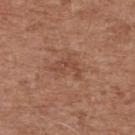Case summary:
* notes · imaged on a skin check; not biopsied
* size · ~4 mm (longest diameter)
* body site · the upper back
* patient · male, in their mid- to late 70s
* acquisition · ~15 mm crop, total-body skin-cancer survey
* lighting · white-light illumination
* TBP lesion metrics · a shape eccentricity near 0.75 and a shape-asymmetry score of about 0.4 (0 = symmetric); a within-lesion color-variation index near 2/10; lesion-presence confidence of about 100/100